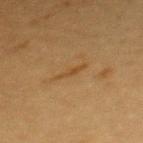Assessment: Recorded during total-body skin imaging; not selected for excision or biopsy. Image and clinical context: Captured under cross-polarized illumination. Cropped from a whole-body photographic skin survey; the tile spans about 15 mm. Located on the mid back. The patient is a female aged 53 to 57. The lesion-visualizer software estimated a footprint of about 3.5 mm², an outline eccentricity of about 0.95 (0 = round, 1 = elongated), and two-axis asymmetry of about 0.3. The software also gave a normalized lesion–skin contrast near 5. The analysis additionally found an automated nevus-likeness rating near 0 out of 100 and a detector confidence of about 95 out of 100 that the crop contains a lesion.